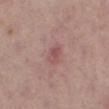The lesion was photographed on a routine skin check and not biopsied; there is no pathology result.
A female subject aged around 60.
Approximately 3 mm at its widest.
The total-body-photography lesion software estimated a nevus-likeness score of about 0/100 and a lesion-detection confidence of about 100/100.
From the leg.
Imaged with white-light lighting.
Cropped from a total-body skin-imaging series; the visible field is about 15 mm.